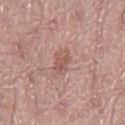Captured during whole-body skin photography for melanoma surveillance; the lesion was not biopsied.
About 3 mm across.
A 15 mm close-up extracted from a 3D total-body photography capture.
This is a white-light tile.
The total-body-photography lesion software estimated a mean CIELAB color near L≈55 a*≈22 b*≈24, roughly 8 lightness units darker than nearby skin, and a normalized lesion–skin contrast near 6.
Located on the left thigh.
A male patient, aged 53 to 57.